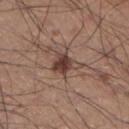• notes · catalogued during a skin exam; not biopsied
• tile lighting · white-light illumination
• location · the left lower leg
• image source · total-body-photography crop, ~15 mm field of view
• subject · male, aged 33 to 37
• lesion size · ~3.5 mm (longest diameter)
• automated lesion analysis · a border-irregularity index near 5/10, internal color variation of about 4 on a 0–10 scale, and radial color variation of about 1.5